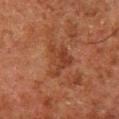No biopsy was performed on this lesion — it was imaged during a full skin examination and was not determined to be concerning.
On the right lower leg.
The tile uses cross-polarized illumination.
A region of skin cropped from a whole-body photographic capture, roughly 15 mm wide.
The patient is a male roughly 80 years of age.
Approximately 4 mm at its widest.